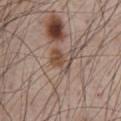No biopsy was performed on this lesion — it was imaged during a full skin examination and was not determined to be concerning. A male subject, roughly 65 years of age. The recorded lesion diameter is about 3.5 mm. Located on the chest. Cropped from a whole-body photographic skin survey; the tile spans about 15 mm.